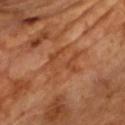The lesion was tiled from a total-body skin photograph and was not biopsied. Imaged with cross-polarized lighting. A male subject in their 60s. The lesion is on the upper back. The recorded lesion diameter is about 5.5 mm. A close-up tile cropped from a whole-body skin photograph, about 15 mm across.